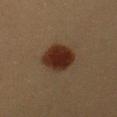follow-up: no biopsy performed (imaged during a skin exam)
image: ~15 mm crop, total-body skin-cancer survey
patient: female, approximately 20 years of age
anatomic site: the left upper arm